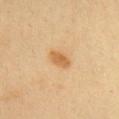The lesion was tiled from a total-body skin photograph and was not biopsied.
Longest diameter approximately 3 mm.
A female patient aged 28–32.
Cropped from a total-body skin-imaging series; the visible field is about 15 mm.
This is a cross-polarized tile.
On the head or neck.
Automated tile analysis of the lesion measured a nevus-likeness score of about 95/100 and a detector confidence of about 100 out of 100 that the crop contains a lesion.